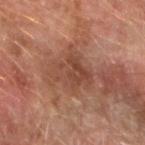<tbp_lesion>
  <biopsy_status>not biopsied; imaged during a skin examination</biopsy_status>
  <automated_metrics>
    <cielab_L>40</cielab_L>
    <cielab_a>20</cielab_a>
    <cielab_b>26</cielab_b>
    <vs_skin_darker_L>7.0</vs_skin_darker_L>
    <vs_skin_contrast_norm>6.0</vs_skin_contrast_norm>
  </automated_metrics>
  <site>right forearm</site>
  <patient>
    <sex>male</sex>
    <age_approx>65</age_approx>
  </patient>
  <image>
    <source>total-body photography crop</source>
    <field_of_view_mm>15</field_of_view_mm>
  </image>
  <lighting>cross-polarized</lighting>
</tbp_lesion>